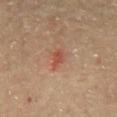Case summary:
- image source: total-body-photography crop, ~15 mm field of view
- location: the chest
- subject: male, aged around 65
- lesion diameter: about 2.5 mm